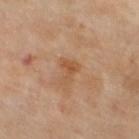follow-up: catalogued during a skin exam; not biopsied
acquisition: ~15 mm crop, total-body skin-cancer survey
subject: female, approximately 70 years of age
tile lighting: cross-polarized
diameter: about 3 mm
automated metrics: an eccentricity of roughly 0.7 and two-axis asymmetry of about 0.6
site: the left lower leg